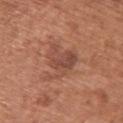<lesion>
<biopsy_status>not biopsied; imaged during a skin examination</biopsy_status>
<lighting>white-light</lighting>
<patient>
  <sex>female</sex>
  <age_approx>65</age_approx>
</patient>
<image>
  <source>total-body photography crop</source>
  <field_of_view_mm>15</field_of_view_mm>
</image>
<lesion_size>
  <long_diameter_mm_approx>4.5</long_diameter_mm_approx>
</lesion_size>
<site>upper back</site>
</lesion>